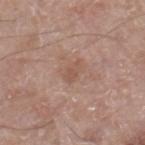  biopsy_status: not biopsied; imaged during a skin examination
  lighting: white-light
  lesion_size:
    long_diameter_mm_approx: 2.5
  automated_metrics:
    cielab_L: 53
    cielab_a: 19
    cielab_b: 27
    vs_skin_darker_L: 7.0
    vs_skin_contrast_norm: 5.0
  patient:
    sex: male
    age_approx: 65
  site: left thigh
  image:
    source: total-body photography crop
    field_of_view_mm: 15